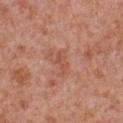No biopsy was performed on this lesion — it was imaged during a full skin examination and was not determined to be concerning.
Located on the chest.
This image is a 15 mm lesion crop taken from a total-body photograph.
A male patient aged 63 to 67.